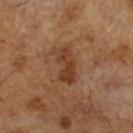biopsy status: total-body-photography surveillance lesion; no biopsy | acquisition: ~15 mm tile from a whole-body skin photo | diameter: about 4.5 mm | subject: male, about 65 years old | illumination: cross-polarized.